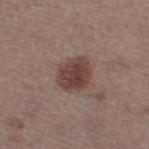No biopsy was performed on this lesion — it was imaged during a full skin examination and was not determined to be concerning. The subject is a female in their 40s. Located on the right thigh. Cropped from a whole-body photographic skin survey; the tile spans about 15 mm. Captured under white-light illumination.